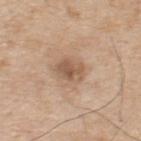| field | value |
|---|---|
| image source | total-body-photography crop, ~15 mm field of view |
| tile lighting | white-light illumination |
| image-analysis metrics | a footprint of about 7 mm² and an eccentricity of roughly 0.75; an average lesion color of about L≈56 a*≈17 b*≈31 (CIELAB), about 10 CIELAB-L* units darker than the surrounding skin, and a normalized lesion–skin contrast near 7; a classifier nevus-likeness of about 5/100 and a lesion-detection confidence of about 100/100 |
| size | ~3.5 mm (longest diameter) |
| subject | male, about 70 years old |
| anatomic site | the upper back |